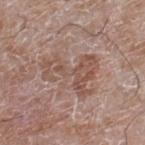- illumination: white-light illumination
- lesion diameter: about 6 mm
- acquisition: 15 mm crop, total-body photography
- location: the right lower leg
- subject: male, aged approximately 60